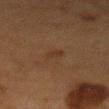Imaged during a routine full-body skin examination; the lesion was not biopsied and no histopathology is available.
Longest diameter approximately 3 mm.
This is a cross-polarized tile.
Located on the mid back.
A 15 mm close-up extracted from a 3D total-body photography capture.
A female subject, approximately 50 years of age.